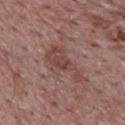Notes:
– workup: imaged on a skin check; not biopsied
– site: the upper back
– lesion size: ~5.5 mm (longest diameter)
– imaging modality: total-body-photography crop, ~15 mm field of view
– subject: male, aged 68–72